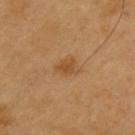workup: no biopsy performed (imaged during a skin exam) | location: the back | automated metrics: a lesion color around L≈48 a*≈21 b*≈39 in CIELAB, about 8 CIELAB-L* units darker than the surrounding skin, and a lesion-to-skin contrast of about 6.5 (normalized; higher = more distinct); a border-irregularity rating of about 3.5/10, internal color variation of about 2.5 on a 0–10 scale, and radial color variation of about 1 | image: 15 mm crop, total-body photography | subject: male, aged 58 to 62.